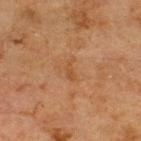Q: Is there a histopathology result?
A: catalogued during a skin exam; not biopsied
Q: Automated lesion metrics?
A: roughly 5 lightness units darker than nearby skin and a lesion-to-skin contrast of about 5 (normalized; higher = more distinct); a classifier nevus-likeness of about 0/100
Q: What is the anatomic site?
A: the upper back
Q: What kind of image is this?
A: ~15 mm tile from a whole-body skin photo
Q: Illumination type?
A: cross-polarized illumination
Q: What is the lesion's diameter?
A: about 2.5 mm
Q: Patient demographics?
A: male, aged approximately 70